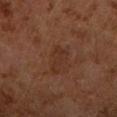Impression: This lesion was catalogued during total-body skin photography and was not selected for biopsy. Image and clinical context: A close-up tile cropped from a whole-body skin photograph, about 15 mm across. The total-body-photography lesion software estimated border irregularity of about 5 on a 0–10 scale and radial color variation of about 0.5. The analysis additionally found a classifier nevus-likeness of about 0/100 and lesion-presence confidence of about 100/100. The tile uses cross-polarized illumination. On the right upper arm. A female subject, about 60 years old. The recorded lesion diameter is about 4 mm.